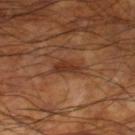Impression: The lesion was tiled from a total-body skin photograph and was not biopsied. Clinical summary: A male patient, aged 58–62. On the right lower leg. Measured at roughly 3 mm in maximum diameter. This is a cross-polarized tile. This image is a 15 mm lesion crop taken from a total-body photograph.